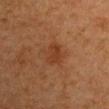workup=total-body-photography surveillance lesion; no biopsy
image source=total-body-photography crop, ~15 mm field of view
subject=male, roughly 65 years of age
lesion size=≈3 mm
body site=the right upper arm
lighting=cross-polarized illumination